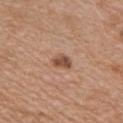Clinical impression:
This lesion was catalogued during total-body skin photography and was not selected for biopsy.
Clinical summary:
A region of skin cropped from a whole-body photographic capture, roughly 15 mm wide. A male subject aged around 65. On the chest. The lesion's longest dimension is about 2.5 mm. This is a white-light tile.